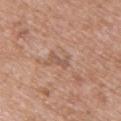follow-up: no biopsy performed (imaged during a skin exam)
automated metrics: a lesion–skin lightness drop of about 8 and a normalized lesion–skin contrast near 5.5; a border-irregularity rating of about 4.5/10, internal color variation of about 0 on a 0–10 scale, and radial color variation of about 0
image source: ~15 mm crop, total-body skin-cancer survey
tile lighting: white-light illumination
subject: female, about 40 years old
lesion size: about 3 mm
anatomic site: the upper back